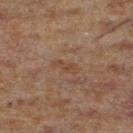The lesion was photographed on a routine skin check and not biopsied; there is no pathology result. Approximately 3 mm at its widest. A male subject in their mid- to late 60s. On the left lower leg. A 15 mm crop from a total-body photograph taken for skin-cancer surveillance.